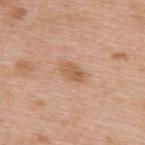Q: Was a biopsy performed?
A: no biopsy performed (imaged during a skin exam)
Q: Lesion location?
A: the upper back
Q: What lighting was used for the tile?
A: white-light
Q: What are the patient's age and sex?
A: female, about 40 years old
Q: What is the lesion's diameter?
A: ≈3 mm
Q: What kind of image is this?
A: ~15 mm tile from a whole-body skin photo
Q: Automated lesion metrics?
A: a lesion–skin lightness drop of about 9 and a normalized lesion–skin contrast near 6.5; border irregularity of about 2 on a 0–10 scale, a color-variation rating of about 2.5/10, and a peripheral color-asymmetry measure near 1; a lesion-detection confidence of about 100/100